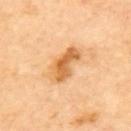Impression: The lesion was photographed on a routine skin check and not biopsied; there is no pathology result. Acquisition and patient details: Automated tile analysis of the lesion measured roughly 13 lightness units darker than nearby skin and a lesion-to-skin contrast of about 8 (normalized; higher = more distinct). A roughly 15 mm field-of-view crop from a total-body skin photograph. Captured under cross-polarized illumination. The lesion is located on the upper back. The lesion's longest dimension is about 5 mm.